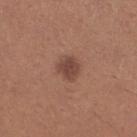notes — no biopsy performed (imaged during a skin exam)
site — the right lower leg
subject — female, aged 53–57
tile lighting — white-light
size — about 2.5 mm
automated lesion analysis — a shape eccentricity near 0.4 and a shape-asymmetry score of about 0.25 (0 = symmetric); a mean CIELAB color near L≈44 a*≈21 b*≈26 and roughly 10 lightness units darker than nearby skin
acquisition — total-body-photography crop, ~15 mm field of view